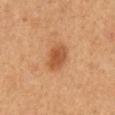workup: no biopsy performed (imaged during a skin exam); patient: male, aged around 60; site: the mid back; image source: 15 mm crop, total-body photography.